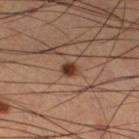Imaged during a routine full-body skin examination; the lesion was not biopsied and no histopathology is available.
The total-body-photography lesion software estimated an outline eccentricity of about 0.6 (0 = round, 1 = elongated) and two-axis asymmetry of about 0.2. The software also gave a normalized lesion–skin contrast near 10.5. It also reported a within-lesion color-variation index near 3/10 and a peripheral color-asymmetry measure near 1. And it measured a nevus-likeness score of about 100/100 and lesion-presence confidence of about 100/100.
Measured at roughly 2 mm in maximum diameter.
A male subject, about 65 years old.
Located on the left lower leg.
A 15 mm close-up tile from a total-body photography series done for melanoma screening.